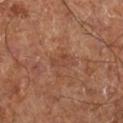biopsy_status: not biopsied; imaged during a skin examination
image:
  source: total-body photography crop
  field_of_view_mm: 15
lighting: cross-polarized
patient:
  age_approx: 65
site: right lower leg
lesion_size:
  long_diameter_mm_approx: 2.5